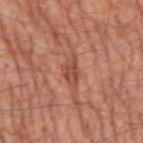The lesion is on the right upper arm.
The total-body-photography lesion software estimated a footprint of about 4.5 mm², a shape eccentricity near 0.85, and a shape-asymmetry score of about 0.35 (0 = symmetric). The analysis additionally found a mean CIELAB color near L≈49 a*≈26 b*≈32, about 9 CIELAB-L* units darker than the surrounding skin, and a lesion-to-skin contrast of about 6.5 (normalized; higher = more distinct). The analysis additionally found a border-irregularity rating of about 3.5/10, a color-variation rating of about 3/10, and radial color variation of about 1.5.
Cropped from a whole-body photographic skin survey; the tile spans about 15 mm.
A male subject, approximately 65 years of age.
The tile uses white-light illumination.